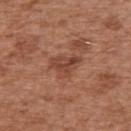image = 15 mm crop, total-body photography; subject = male, approximately 75 years of age; location = the right upper arm.